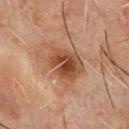image = 15 mm crop, total-body photography
illumination = cross-polarized illumination
size = about 6 mm
site = the front of the torso
patient = male, aged approximately 60